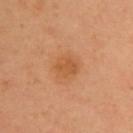- biopsy status: no biopsy performed (imaged during a skin exam)
- lesion size: ~2.5 mm (longest diameter)
- image-analysis metrics: a lesion color around L≈47 a*≈22 b*≈36 in CIELAB and a normalized lesion–skin contrast near 6; a border-irregularity index near 2/10, a color-variation rating of about 2/10, and a peripheral color-asymmetry measure near 0.5; an automated nevus-likeness rating near 5 out of 100 and a detector confidence of about 100 out of 100 that the crop contains a lesion
- tile lighting: cross-polarized illumination
- anatomic site: the chest
- subject: female, about 60 years old
- acquisition: total-body-photography crop, ~15 mm field of view